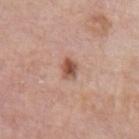Imaged during a routine full-body skin examination; the lesion was not biopsied and no histopathology is available.
A 15 mm crop from a total-body photograph taken for skin-cancer surveillance.
The patient is a male about 75 years old.
On the left upper arm.